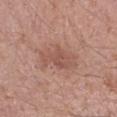lesion_size:
  long_diameter_mm_approx: 4.5
site: right forearm
automated_metrics:
  color_variation_0_10: 2.5
  peripheral_color_asymmetry: 1.0
lighting: white-light
patient:
  sex: female
  age_approx: 40
image:
  source: total-body photography crop
  field_of_view_mm: 15